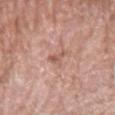Q: Where on the body is the lesion?
A: the arm
Q: What is the imaging modality?
A: ~15 mm tile from a whole-body skin photo
Q: What are the patient's age and sex?
A: male, roughly 80 years of age